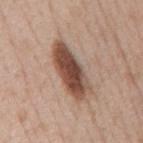Recorded during total-body skin imaging; not selected for excision or biopsy. On the mid back. Captured under white-light illumination. A male subject aged 48–52. A lesion tile, about 15 mm wide, cut from a 3D total-body photograph. The lesion's longest dimension is about 7 mm.